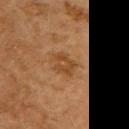Findings:
• biopsy status · no biopsy performed (imaged during a skin exam)
• tile lighting · cross-polarized
• patient · female, aged around 60
• acquisition · total-body-photography crop, ~15 mm field of view
• automated lesion analysis · a border-irregularity index near 3.5/10, a color-variation rating of about 3/10, and peripheral color asymmetry of about 1
• location · the back